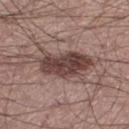{
  "biopsy_status": "not biopsied; imaged during a skin examination",
  "site": "left thigh",
  "patient": {
    "sex": "male",
    "age_approx": 55
  },
  "lighting": "white-light",
  "image": {
    "source": "total-body photography crop",
    "field_of_view_mm": 15
  },
  "lesion_size": {
    "long_diameter_mm_approx": 6.5
  }
}